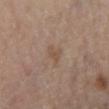Impression: Part of a total-body skin-imaging series; this lesion was reviewed on a skin check and was not flagged for biopsy. Acquisition and patient details: A female patient, aged 58 to 62. The lesion-visualizer software estimated a mean CIELAB color near L≈50 a*≈16 b*≈26 and a lesion-to-skin contrast of about 5 (normalized; higher = more distinct). This is a cross-polarized tile. A roughly 15 mm field-of-view crop from a total-body skin photograph. On the left lower leg. Approximately 3 mm at its widest.